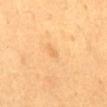<lesion>
  <biopsy_status>not biopsied; imaged during a skin examination</biopsy_status>
  <site>mid back</site>
  <image>
    <source>total-body photography crop</source>
    <field_of_view_mm>15</field_of_view_mm>
  </image>
  <patient>
    <sex>female</sex>
    <age_approx>40</age_approx>
  </patient>
  <lesion_size>
    <long_diameter_mm_approx>1.0</long_diameter_mm_approx>
  </lesion_size>
  <automated_metrics>
    <border_irregularity_0_10>2.5</border_irregularity_0_10>
    <color_variation_0_10>0.0</color_variation_0_10>
    <peripheral_color_asymmetry>0.0</peripheral_color_asymmetry>
    <nevus_likeness_0_100>0</nevus_likeness_0_100>
  </automated_metrics>
  <lighting>cross-polarized</lighting>
</lesion>